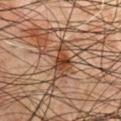| key | value |
|---|---|
| biopsy status | imaged on a skin check; not biopsied |
| location | the chest |
| imaging modality | 15 mm crop, total-body photography |
| patient | male, roughly 65 years of age |
| diameter | about 4 mm |